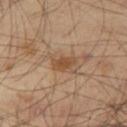biopsy status: no biopsy performed (imaged during a skin exam) | lighting: cross-polarized illumination | imaging modality: ~15 mm tile from a whole-body skin photo | body site: the leg | patient: male, in their 40s | image-analysis metrics: a lesion area of about 4.5 mm², an outline eccentricity of about 0.8 (0 = round, 1 = elongated), and a shape-asymmetry score of about 0.3 (0 = symmetric); a lesion color around L≈49 a*≈19 b*≈33 in CIELAB and about 9 CIELAB-L* units darker than the surrounding skin; border irregularity of about 3 on a 0–10 scale, a within-lesion color-variation index near 2/10, and peripheral color asymmetry of about 0.5; a classifier nevus-likeness of about 35/100 and lesion-presence confidence of about 100/100.